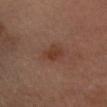Clinical impression: The lesion was tiled from a total-body skin photograph and was not biopsied. Context: A roughly 15 mm field-of-view crop from a total-body skin photograph. From the head or neck. The recorded lesion diameter is about 2.5 mm. Automated image analysis of the tile measured a footprint of about 4.5 mm² and a symmetry-axis asymmetry near 0.3. It also reported a border-irregularity index near 2.5/10 and a color-variation rating of about 3/10. And it measured an automated nevus-likeness rating near 50 out of 100 and lesion-presence confidence of about 100/100. A female patient about 35 years old.